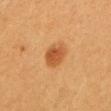Findings:
* workup: no biopsy performed (imaged during a skin exam)
* lighting: cross-polarized illumination
* subject: female, in their 30s
* anatomic site: the chest
* acquisition: ~15 mm tile from a whole-body skin photo
* diameter: ~4 mm (longest diameter)